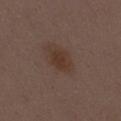Q: Patient demographics?
A: female, aged around 30
Q: What is the anatomic site?
A: the mid back
Q: How was this image acquired?
A: 15 mm crop, total-body photography